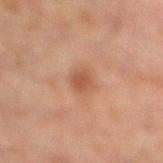Findings:
- follow-up — total-body-photography surveillance lesion; no biopsy
- patient — male, aged 28 to 32
- TBP lesion metrics — a footprint of about 4.5 mm², an eccentricity of roughly 0.7, and a shape-asymmetry score of about 0.15 (0 = symmetric); internal color variation of about 1.5 on a 0–10 scale and a peripheral color-asymmetry measure near 0.5
- site — the right lower leg
- image source — ~15 mm tile from a whole-body skin photo
- illumination — cross-polarized